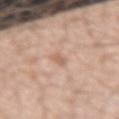Case summary:
• follow-up — catalogued during a skin exam; not biopsied
• anatomic site — the arm
• subject — male, about 45 years old
• imaging modality — total-body-photography crop, ~15 mm field of view
• lighting — white-light illumination
• diameter — about 2.5 mm